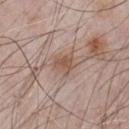Assessment:
The lesion was tiled from a total-body skin photograph and was not biopsied.
Image and clinical context:
This is a white-light tile. Located on the chest. Longest diameter approximately 3 mm. The patient is a male about 65 years old. A region of skin cropped from a whole-body photographic capture, roughly 15 mm wide.